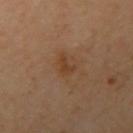This lesion was catalogued during total-body skin photography and was not selected for biopsy. A male patient, in their mid- to late 60s. This image is a 15 mm lesion crop taken from a total-body photograph. Captured under cross-polarized illumination. Longest diameter approximately 3 mm. The lesion is on the right upper arm.